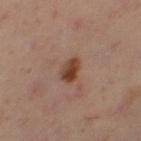This lesion was catalogued during total-body skin photography and was not selected for biopsy.
A 15 mm close-up extracted from a 3D total-body photography capture.
Approximately 3 mm at its widest.
A female subject aged around 40.
Automated image analysis of the tile measured an eccentricity of roughly 0.75 and a shape-asymmetry score of about 0.2 (0 = symmetric). The analysis additionally found about 12 CIELAB-L* units darker than the surrounding skin and a normalized lesion–skin contrast near 10. And it measured border irregularity of about 2 on a 0–10 scale and radial color variation of about 1.5.
The lesion is on the leg.